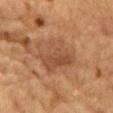The lesion was photographed on a routine skin check and not biopsied; there is no pathology result. Cropped from a whole-body photographic skin survey; the tile spans about 15 mm. A male patient, in their mid- to late 80s or older. The lesion-visualizer software estimated an average lesion color of about L≈42 a*≈20 b*≈30 (CIELAB). The software also gave a border-irregularity rating of about 3/10, internal color variation of about 4 on a 0–10 scale, and radial color variation of about 1.5. And it measured a classifier nevus-likeness of about 0/100. This is a cross-polarized tile. On the chest. The recorded lesion diameter is about 5 mm.